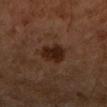Part of a total-body skin-imaging series; this lesion was reviewed on a skin check and was not flagged for biopsy.
A female patient aged 58 to 62.
Approximately 3 mm at its widest.
A lesion tile, about 15 mm wide, cut from a 3D total-body photograph.
On the arm.